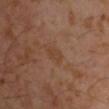<tbp_lesion>
  <biopsy_status>not biopsied; imaged during a skin examination</biopsy_status>
  <image>
    <source>total-body photography crop</source>
    <field_of_view_mm>15</field_of_view_mm>
  </image>
  <site>arm</site>
  <patient>
    <sex>male</sex>
    <age_approx>30</age_approx>
  </patient>
  <lesion_size>
    <long_diameter_mm_approx>2.5</long_diameter_mm_approx>
  </lesion_size>
  <lighting>cross-polarized</lighting>
</tbp_lesion>